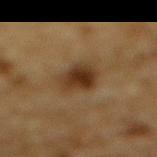image source=~15 mm tile from a whole-body skin photo | anatomic site=the back | automated metrics=a footprint of about 11 mm² and an eccentricity of roughly 0.85; a classifier nevus-likeness of about 95/100 and lesion-presence confidence of about 100/100 | patient=male, about 85 years old or older | illumination=cross-polarized illumination.